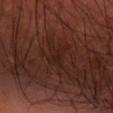notes — imaged on a skin check; not biopsied
anatomic site — the arm
image — ~15 mm tile from a whole-body skin photo
patient — male, in their 70s